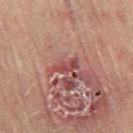follow-up = no biopsy performed (imaged during a skin exam) | patient = female, approximately 80 years of age | acquisition = ~15 mm tile from a whole-body skin photo | illumination = cross-polarized | TBP lesion metrics = a footprint of about 4 mm², an eccentricity of roughly 0.9, and a symmetry-axis asymmetry near 0.35; a classifier nevus-likeness of about 0/100 and lesion-presence confidence of about 65/100 | anatomic site = the left leg.